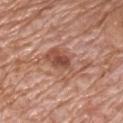Q: Is there a histopathology result?
A: total-body-photography surveillance lesion; no biopsy
Q: How was the tile lit?
A: white-light illumination
Q: Automated lesion metrics?
A: a footprint of about 10 mm²; a nevus-likeness score of about 0/100 and a lesion-detection confidence of about 100/100
Q: What is the imaging modality?
A: total-body-photography crop, ~15 mm field of view
Q: Lesion location?
A: the chest
Q: Who is the patient?
A: male, in their 70s
Q: How large is the lesion?
A: about 4.5 mm